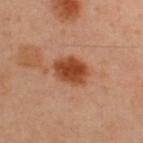Part of a total-body skin-imaging series; this lesion was reviewed on a skin check and was not flagged for biopsy. The lesion is located on the back. A 15 mm close-up extracted from a 3D total-body photography capture. A male patient, in their mid-40s.